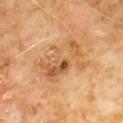Q: Was this lesion biopsied?
A: catalogued during a skin exam; not biopsied
Q: How was this image acquired?
A: total-body-photography crop, ~15 mm field of view
Q: How was the tile lit?
A: cross-polarized
Q: Lesion size?
A: ~6 mm (longest diameter)
Q: Patient demographics?
A: male, in their 60s
Q: Lesion location?
A: the chest
Q: What did automated image analysis measure?
A: a footprint of about 16 mm², an outline eccentricity of about 0.8 (0 = round, 1 = elongated), and two-axis asymmetry of about 0.4